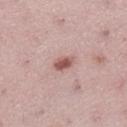Impression: Captured during whole-body skin photography for melanoma surveillance; the lesion was not biopsied. Context: A female subject in their mid-20s. A close-up tile cropped from a whole-body skin photograph, about 15 mm across. On the left lower leg.